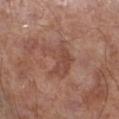<tbp_lesion>
  <biopsy_status>not biopsied; imaged during a skin examination</biopsy_status>
  <lesion_size>
    <long_diameter_mm_approx>4.5</long_diameter_mm_approx>
  </lesion_size>
  <image>
    <source>total-body photography crop</source>
    <field_of_view_mm>15</field_of_view_mm>
  </image>
  <patient>
    <sex>male</sex>
    <age_approx>70</age_approx>
  </patient>
  <site>left lower leg</site>
</tbp_lesion>